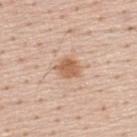The lesion was photographed on a routine skin check and not biopsied; there is no pathology result. The lesion's longest dimension is about 3 mm. This is a white-light tile. A male patient, about 60 years old. Cropped from a total-body skin-imaging series; the visible field is about 15 mm. The total-body-photography lesion software estimated a detector confidence of about 100 out of 100 that the crop contains a lesion. Located on the back.